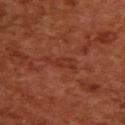| field | value |
|---|---|
| biopsy status | no biopsy performed (imaged during a skin exam) |
| lighting | cross-polarized |
| size | ≈4 mm |
| imaging modality | ~15 mm crop, total-body skin-cancer survey |
| automated metrics | a border-irregularity rating of about 6/10, a within-lesion color-variation index near 1/10, and radial color variation of about 0 |
| body site | the upper back |
| subject | female, in their mid-50s |